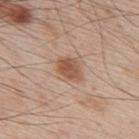workup: catalogued during a skin exam; not biopsied | body site: the upper back | diameter: ~3.5 mm (longest diameter) | subject: male, aged 63–67 | lighting: white-light illumination | image source: total-body-photography crop, ~15 mm field of view.